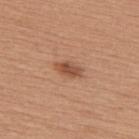<tbp_lesion>
  <biopsy_status>not biopsied; imaged during a skin examination</biopsy_status>
  <patient>
    <sex>female</sex>
    <age_approx>45</age_approx>
  </patient>
  <image>
    <source>total-body photography crop</source>
    <field_of_view_mm>15</field_of_view_mm>
  </image>
  <site>upper back</site>
  <lighting>white-light</lighting>
  <lesion_size>
    <long_diameter_mm_approx>3.5</long_diameter_mm_approx>
  </lesion_size>
</tbp_lesion>